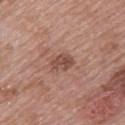biopsy status: no biopsy performed (imaged during a skin exam).